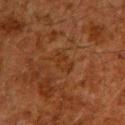The lesion was tiled from a total-body skin photograph and was not biopsied. This image is a 15 mm lesion crop taken from a total-body photograph. The total-body-photography lesion software estimated an average lesion color of about L≈26 a*≈19 b*≈28 (CIELAB) and about 4 CIELAB-L* units darker than the surrounding skin. It also reported a border-irregularity index near 2.5/10, internal color variation of about 2 on a 0–10 scale, and a peripheral color-asymmetry measure near 0.5. The software also gave a classifier nevus-likeness of about 0/100 and lesion-presence confidence of about 95/100. About 3 mm across. Located on the upper back. Imaged with cross-polarized lighting. A male subject, aged 58–62.